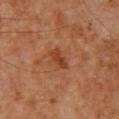The lesion was tiled from a total-body skin photograph and was not biopsied.
The lesion is on the upper back.
The recorded lesion diameter is about 3 mm.
Cropped from a whole-body photographic skin survey; the tile spans about 15 mm.
Automated tile analysis of the lesion measured a lesion area of about 3.5 mm², an eccentricity of roughly 0.85, and a symmetry-axis asymmetry near 0.3. The analysis additionally found a lesion color around L≈36 a*≈24 b*≈32 in CIELAB, about 8 CIELAB-L* units darker than the surrounding skin, and a normalized lesion–skin contrast near 7.
A male subject, aged 58–62.